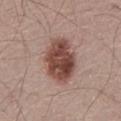| key | value |
|---|---|
| image source | total-body-photography crop, ~15 mm field of view |
| anatomic site | the abdomen |
| illumination | white-light illumination |
| subject | male, about 60 years old |
| size | about 6 mm |